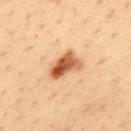No biopsy was performed on this lesion — it was imaged during a full skin examination and was not determined to be concerning.
About 4.5 mm across.
The subject is a male in their mid- to late 30s.
The lesion is located on the upper back.
A 15 mm close-up extracted from a 3D total-body photography capture.
Imaged with cross-polarized lighting.